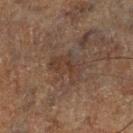Q: What is the lesion's diameter?
A: ~4 mm (longest diameter)
Q: How was the tile lit?
A: cross-polarized illumination
Q: Lesion location?
A: the left lower leg
Q: Patient demographics?
A: male, in their mid-60s
Q: How was this image acquired?
A: 15 mm crop, total-body photography